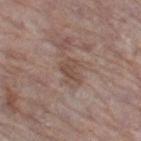- workup · catalogued during a skin exam; not biopsied
- body site · the left thigh
- illumination · white-light illumination
- patient · female, in their mid- to late 80s or older
- lesion diameter · ≈3 mm
- imaging modality · ~15 mm tile from a whole-body skin photo